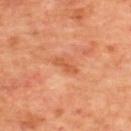Assessment:
No biopsy was performed on this lesion — it was imaged during a full skin examination and was not determined to be concerning.
Background:
From the upper back. A 15 mm crop from a total-body photograph taken for skin-cancer surveillance. Captured under cross-polarized illumination. A female subject, aged around 45.